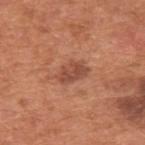lighting: white-light
automated_metrics:
  cielab_L: 49
  cielab_a: 26
  cielab_b: 32
  vs_skin_darker_L: 10.0
  border_irregularity_0_10: 3.0
  lesion_detection_confidence_0_100: 100
image:
  source: total-body photography crop
  field_of_view_mm: 15
site: back
patient:
  sex: male
  age_approx: 65